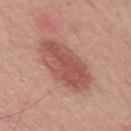Clinical impression:
This lesion was catalogued during total-body skin photography and was not selected for biopsy.
Acquisition and patient details:
Located on the mid back. A male patient, aged around 65. This is a white-light tile. A 15 mm close-up extracted from a 3D total-body photography capture. The total-body-photography lesion software estimated an area of roughly 23 mm² and two-axis asymmetry of about 0.15. The lesion's longest dimension is about 7.5 mm.